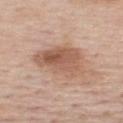Q: Was a biopsy performed?
A: imaged on a skin check; not biopsied
Q: Who is the patient?
A: male, aged approximately 60
Q: How was this image acquired?
A: ~15 mm crop, total-body skin-cancer survey
Q: What did automated image analysis measure?
A: a lesion area of about 19 mm², an outline eccentricity of about 0.75 (0 = round, 1 = elongated), and a shape-asymmetry score of about 0.3 (0 = symmetric)
Q: What is the lesion's diameter?
A: about 6.5 mm
Q: What is the anatomic site?
A: the upper back
Q: How was the tile lit?
A: white-light illumination